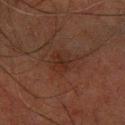Context:
Automated image analysis of the tile measured a lesion color around L≈21 a*≈16 b*≈21 in CIELAB, about 4 CIELAB-L* units darker than the surrounding skin, and a normalized lesion–skin contrast near 5.5. The software also gave a border-irregularity index near 2.5/10 and internal color variation of about 2.5 on a 0–10 scale. The analysis additionally found a classifier nevus-likeness of about 0/100 and a detector confidence of about 100 out of 100 that the crop contains a lesion. Longest diameter approximately 3 mm. A male subject, aged around 80. A 15 mm close-up extracted from a 3D total-body photography capture. Located on the left forearm. The tile uses cross-polarized illumination.